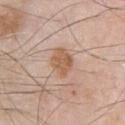The lesion was tiled from a total-body skin photograph and was not biopsied. A 15 mm crop from a total-body photograph taken for skin-cancer surveillance. Located on the chest. The tile uses white-light illumination. Approximately 3.5 mm at its widest. The total-body-photography lesion software estimated border irregularity of about 2.5 on a 0–10 scale, a color-variation rating of about 2.5/10, and peripheral color asymmetry of about 1. The software also gave a detector confidence of about 100 out of 100 that the crop contains a lesion. A male subject, aged 53 to 57.